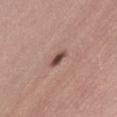This lesion was catalogued during total-body skin photography and was not selected for biopsy.
The patient is a female roughly 25 years of age.
The tile uses white-light illumination.
A 15 mm close-up extracted from a 3D total-body photography capture.
The lesion's longest dimension is about 3 mm.
Located on the abdomen.
The total-body-photography lesion software estimated a lesion area of about 3 mm², an eccentricity of roughly 0.85, and a symmetry-axis asymmetry near 0.25. The software also gave a lesion color around L≈48 a*≈21 b*≈24 in CIELAB, roughly 14 lightness units darker than nearby skin, and a normalized border contrast of about 9.5. The software also gave radial color variation of about 0.5.